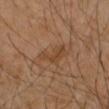Q: Is there a histopathology result?
A: catalogued during a skin exam; not biopsied
Q: What kind of image is this?
A: 15 mm crop, total-body photography
Q: What are the patient's age and sex?
A: female, in their mid-40s
Q: Lesion location?
A: the arm